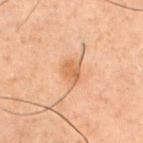Q: Was a biopsy performed?
A: no biopsy performed (imaged during a skin exam)
Q: What is the imaging modality?
A: ~15 mm tile from a whole-body skin photo
Q: What are the patient's age and sex?
A: male, aged approximately 60
Q: What is the anatomic site?
A: the chest
Q: How large is the lesion?
A: ≈3.5 mm